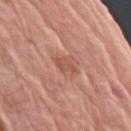Q: Was this lesion biopsied?
A: no biopsy performed (imaged during a skin exam)
Q: Lesion size?
A: ~2.5 mm (longest diameter)
Q: What is the anatomic site?
A: the right upper arm
Q: Patient demographics?
A: male, aged around 80
Q: How was this image acquired?
A: total-body-photography crop, ~15 mm field of view
Q: How was the tile lit?
A: white-light illumination
Q: What did automated image analysis measure?
A: a lesion area of about 3.5 mm², a shape eccentricity near 0.8, and a symmetry-axis asymmetry near 0.35; roughly 8 lightness units darker than nearby skin and a lesion-to-skin contrast of about 6 (normalized; higher = more distinct); peripheral color asymmetry of about 0.5